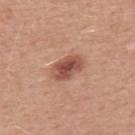Q: Was this lesion biopsied?
A: imaged on a skin check; not biopsied
Q: What is the anatomic site?
A: the upper back
Q: Patient demographics?
A: male, roughly 50 years of age
Q: What is the imaging modality?
A: ~15 mm tile from a whole-body skin photo